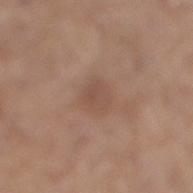<lesion>
  <biopsy_status>not biopsied; imaged during a skin examination</biopsy_status>
  <image>
    <source>total-body photography crop</source>
    <field_of_view_mm>15</field_of_view_mm>
  </image>
  <lesion_size>
    <long_diameter_mm_approx>3.0</long_diameter_mm_approx>
  </lesion_size>
  <lighting>white-light</lighting>
  <patient>
    <sex>male</sex>
    <age_approx>65</age_approx>
  </patient>
  <site>right lower leg</site>
</lesion>